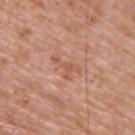follow-up=imaged on a skin check; not biopsied
acquisition=total-body-photography crop, ~15 mm field of view
lesion diameter=about 4 mm
patient=male, aged 73–77
site=the upper back
tile lighting=white-light illumination